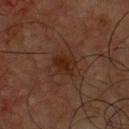| key | value |
|---|---|
| follow-up | catalogued during a skin exam; not biopsied |
| imaging modality | 15 mm crop, total-body photography |
| location | the chest |
| TBP lesion metrics | an average lesion color of about L≈23 a*≈19 b*≈25 (CIELAB) and a normalized border contrast of about 8; a border-irregularity index near 2.5/10 and internal color variation of about 2 on a 0–10 scale; an automated nevus-likeness rating near 25 out of 100 and lesion-presence confidence of about 100/100 |
| patient | male, aged 58 to 62 |
| lesion diameter | ≈3 mm |
| lighting | cross-polarized |